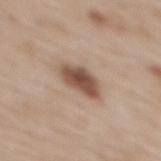The lesion was tiled from a total-body skin photograph and was not biopsied. A female patient, approximately 50 years of age. The recorded lesion diameter is about 4.5 mm. Automated tile analysis of the lesion measured a border-irregularity rating of about 2/10, a within-lesion color-variation index near 4/10, and peripheral color asymmetry of about 1. The analysis additionally found a nevus-likeness score of about 90/100 and lesion-presence confidence of about 100/100. A region of skin cropped from a whole-body photographic capture, roughly 15 mm wide. From the upper back.